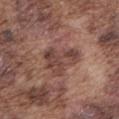workup=catalogued during a skin exam; not biopsied | image=~15 mm tile from a whole-body skin photo | lighting=white-light illumination | lesion diameter=≈5 mm | location=the mid back | TBP lesion metrics=an average lesion color of about L≈44 a*≈20 b*≈23 (CIELAB) and roughly 10 lightness units darker than nearby skin | patient=male, aged 73 to 77.